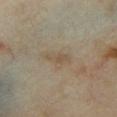Part of a total-body skin-imaging series; this lesion was reviewed on a skin check and was not flagged for biopsy. The lesion is on the front of the torso. A close-up tile cropped from a whole-body skin photograph, about 15 mm across. A female patient, about 35 years old. Longest diameter approximately 3.5 mm. This is a cross-polarized tile.